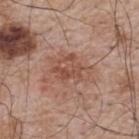This lesion was catalogued during total-body skin photography and was not selected for biopsy. A roughly 15 mm field-of-view crop from a total-body skin photograph. From the back. A male subject in their mid- to late 60s.